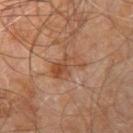| key | value |
|---|---|
| follow-up | imaged on a skin check; not biopsied |
| anatomic site | the leg |
| patient | male, aged around 60 |
| tile lighting | cross-polarized |
| imaging modality | ~15 mm crop, total-body skin-cancer survey |